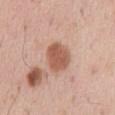Q: Was a biopsy performed?
A: total-body-photography surveillance lesion; no biopsy
Q: How was this image acquired?
A: ~15 mm tile from a whole-body skin photo
Q: Automated lesion metrics?
A: an area of roughly 10 mm² and a symmetry-axis asymmetry near 0.15; a detector confidence of about 100 out of 100 that the crop contains a lesion
Q: Where on the body is the lesion?
A: the abdomen
Q: Patient demographics?
A: male, about 55 years old
Q: What is the lesion's diameter?
A: ~4 mm (longest diameter)
Q: What lighting was used for the tile?
A: white-light illumination